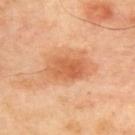Q: Was this lesion biopsied?
A: no biopsy performed (imaged during a skin exam)
Q: What is the lesion's diameter?
A: about 5 mm
Q: Patient demographics?
A: female, aged around 40
Q: How was the tile lit?
A: cross-polarized illumination
Q: What is the imaging modality?
A: total-body-photography crop, ~15 mm field of view
Q: Where on the body is the lesion?
A: the upper back